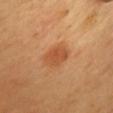Assessment:
Imaged during a routine full-body skin examination; the lesion was not biopsied and no histopathology is available.
Context:
On the front of the torso. A roughly 15 mm field-of-view crop from a total-body skin photograph. A male subject, approximately 65 years of age.